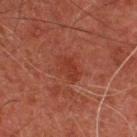- workup — imaged on a skin check; not biopsied
- imaging modality — total-body-photography crop, ~15 mm field of view
- lesion diameter — ≈3.5 mm
- anatomic site — the upper back
- image-analysis metrics — a shape eccentricity near 0.75 and a symmetry-axis asymmetry near 0.2; a border-irregularity rating of about 2.5/10, a color-variation rating of about 1.5/10, and radial color variation of about 0.5; lesion-presence confidence of about 100/100
- patient — male, in their mid-40s